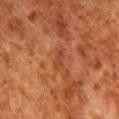image source=15 mm crop, total-body photography | body site=the right lower leg | patient=male, aged 78 to 82 | lesion diameter=≈3 mm | illumination=cross-polarized.